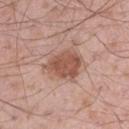| feature | finding |
|---|---|
| follow-up | total-body-photography surveillance lesion; no biopsy |